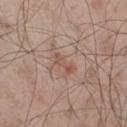Case summary:
- workup — imaged on a skin check; not biopsied
- TBP lesion metrics — an area of roughly 2.5 mm², an eccentricity of roughly 0.95, and two-axis asymmetry of about 0.4; a classifier nevus-likeness of about 10/100 and lesion-presence confidence of about 100/100
- lighting — white-light illumination
- imaging modality — ~15 mm crop, total-body skin-cancer survey
- anatomic site — the right thigh
- patient — male, aged around 55
- lesion diameter — about 2.5 mm